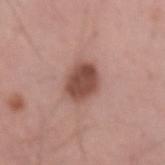illumination=white-light | TBP lesion metrics=an eccentricity of roughly 0.55 and a symmetry-axis asymmetry near 0.2; a classifier nevus-likeness of about 75/100 and a lesion-detection confidence of about 100/100 | lesion size=about 3.5 mm | location=the left forearm | image source=total-body-photography crop, ~15 mm field of view | subject=male, aged 58 to 62.